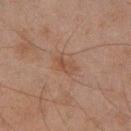Findings:
– biopsy status — no biopsy performed (imaged during a skin exam)
– site — the left lower leg
– imaging modality — ~15 mm tile from a whole-body skin photo
– subject — male, approximately 45 years of age
– tile lighting — cross-polarized
– image-analysis metrics — peripheral color asymmetry of about 0.5; an automated nevus-likeness rating near 0 out of 100 and a detector confidence of about 100 out of 100 that the crop contains a lesion